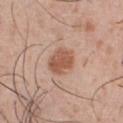No biopsy was performed on this lesion — it was imaged during a full skin examination and was not determined to be concerning. The patient is a male roughly 30 years of age. A 15 mm close-up tile from a total-body photography series done for melanoma screening. From the chest.